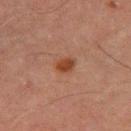The lesion was photographed on a routine skin check and not biopsied; there is no pathology result.
Located on the left thigh.
Cropped from a whole-body photographic skin survey; the tile spans about 15 mm.
Approximately 2.5 mm at its widest.
Imaged with cross-polarized lighting.
An algorithmic analysis of the crop reported a shape eccentricity near 0.6. And it measured a detector confidence of about 100 out of 100 that the crop contains a lesion.
The subject is a male aged 68–72.